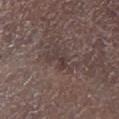Impression:
This lesion was catalogued during total-body skin photography and was not selected for biopsy.
Background:
This is a white-light tile. Located on the left lower leg. A roughly 15 mm field-of-view crop from a total-body skin photograph. Longest diameter approximately 2.5 mm. A male patient in their 70s.